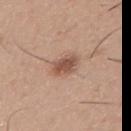| field | value |
|---|---|
| image | ~15 mm tile from a whole-body skin photo |
| subject | male, approximately 30 years of age |
| body site | the chest |